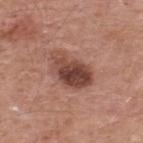notes: no biopsy performed (imaged during a skin exam)
automated metrics: an area of roughly 13 mm² and a shape-asymmetry score of about 0.25 (0 = symmetric); about 14 CIELAB-L* units darker than the surrounding skin; an automated nevus-likeness rating near 60 out of 100 and a detector confidence of about 100 out of 100 that the crop contains a lesion
diameter: ~5.5 mm (longest diameter)
image source: 15 mm crop, total-body photography
illumination: white-light illumination
location: the upper back
subject: male, aged approximately 55